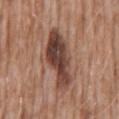{
  "biopsy_status": "not biopsied; imaged during a skin examination",
  "lesion_size": {
    "long_diameter_mm_approx": 8.5
  },
  "image": {
    "source": "total-body photography crop",
    "field_of_view_mm": 15
  },
  "patient": {
    "sex": "male",
    "age_approx": 60
  },
  "lighting": "white-light",
  "automated_metrics": {
    "area_mm2_approx": 20.0,
    "eccentricity": 0.9,
    "cielab_L": 43,
    "cielab_a": 20,
    "cielab_b": 25,
    "vs_skin_darker_L": 15.0
  },
  "site": "abdomen"
}